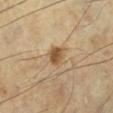notes: total-body-photography surveillance lesion; no biopsy | image: ~15 mm crop, total-body skin-cancer survey | subject: male, aged approximately 55 | lesion size: about 2.5 mm | anatomic site: the leg.